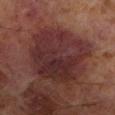Recorded during total-body skin imaging; not selected for excision or biopsy.